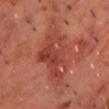* workup · catalogued during a skin exam; not biopsied
* site · the chest
* image-analysis metrics · a footprint of about 22 mm² and a shape eccentricity near 0.9; a lesion color around L≈42 a*≈30 b*≈30 in CIELAB, roughly 8 lightness units darker than nearby skin, and a lesion-to-skin contrast of about 6 (normalized; higher = more distinct)
* image source · ~15 mm tile from a whole-body skin photo
* subject · male, roughly 70 years of age
* diameter · ≈10 mm